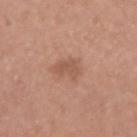The lesion was tiled from a total-body skin photograph and was not biopsied. This image is a 15 mm lesion crop taken from a total-body photograph. The patient is a female roughly 45 years of age. This is a white-light tile. Located on the right upper arm. The recorded lesion diameter is about 3 mm.